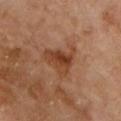Notes:
• workup: imaged on a skin check; not biopsied
• illumination: cross-polarized illumination
• subject: male, about 70 years old
• site: the chest
• automated metrics: a shape eccentricity near 0.45 and a symmetry-axis asymmetry near 0.45; a mean CIELAB color near L≈41 a*≈22 b*≈33; a border-irregularity rating of about 5.5/10 and radial color variation of about 2
• image source: 15 mm crop, total-body photography